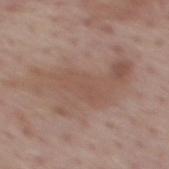Clinical impression: No biopsy was performed on this lesion — it was imaged during a full skin examination and was not determined to be concerning. Context: The lesion is on the mid back. This is a white-light tile. Cropped from a whole-body photographic skin survey; the tile spans about 15 mm. A male patient aged 73–77. About 10 mm across. Automated image analysis of the tile measured a footprint of about 28 mm² and an outline eccentricity of about 0.9 (0 = round, 1 = elongated). And it measured an automated nevus-likeness rating near 0 out of 100 and lesion-presence confidence of about 95/100.